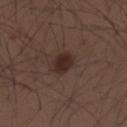This lesion was catalogued during total-body skin photography and was not selected for biopsy.